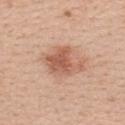Clinical impression:
No biopsy was performed on this lesion — it was imaged during a full skin examination and was not determined to be concerning.
Acquisition and patient details:
A male patient roughly 35 years of age. From the mid back. A region of skin cropped from a whole-body photographic capture, roughly 15 mm wide.